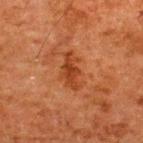| key | value |
|---|---|
| follow-up | no biopsy performed (imaged during a skin exam) |
| image source | ~15 mm tile from a whole-body skin photo |
| body site | the upper back |
| patient | male, aged 58 to 62 |